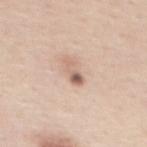| field | value |
|---|---|
| diameter | about 3 mm |
| imaging modality | total-body-photography crop, ~15 mm field of view |
| illumination | white-light illumination |
| patient | female, in their mid- to late 40s |
| TBP lesion metrics | an area of roughly 3.5 mm², a shape eccentricity near 0.95, and two-axis asymmetry of about 0.4; a lesion color around L≈62 a*≈18 b*≈27 in CIELAB; border irregularity of about 5 on a 0–10 scale and radial color variation of about 0.5; a nevus-likeness score of about 0/100 |
| site | the upper back |